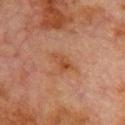The lesion was tiled from a total-body skin photograph and was not biopsied. The tile uses cross-polarized illumination. A male patient approximately 80 years of age. The recorded lesion diameter is about 3 mm. A 15 mm close-up tile from a total-body photography series done for melanoma screening. From the chest.